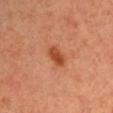The lesion was tiled from a total-body skin photograph and was not biopsied.
The tile uses cross-polarized illumination.
A male patient in their mid- to late 30s.
About 3 mm across.
A 15 mm crop from a total-body photograph taken for skin-cancer surveillance.
Located on the left upper arm.
The total-body-photography lesion software estimated a footprint of about 5 mm², an outline eccentricity of about 0.8 (0 = round, 1 = elongated), and two-axis asymmetry of about 0.15. The analysis additionally found an average lesion color of about L≈47 a*≈28 b*≈37 (CIELAB), roughly 10 lightness units darker than nearby skin, and a lesion-to-skin contrast of about 8 (normalized; higher = more distinct). The analysis additionally found border irregularity of about 2 on a 0–10 scale, a color-variation rating of about 2.5/10, and radial color variation of about 1.